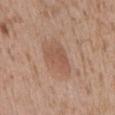Impression: No biopsy was performed on this lesion — it was imaged during a full skin examination and was not determined to be concerning. Clinical summary: The tile uses white-light illumination. The patient is a male in their mid- to late 40s. Located on the abdomen. The lesion-visualizer software estimated an eccentricity of roughly 0.8. The recorded lesion diameter is about 5 mm. A 15 mm close-up extracted from a 3D total-body photography capture.